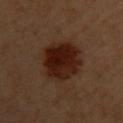Imaged during a routine full-body skin examination; the lesion was not biopsied and no histopathology is available. A female subject aged around 60. A roughly 15 mm field-of-view crop from a total-body skin photograph. On the chest. Longest diameter approximately 6 mm.